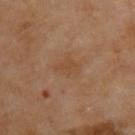Clinical impression:
Imaged during a routine full-body skin examination; the lesion was not biopsied and no histopathology is available.
Background:
Captured under cross-polarized illumination. A male patient, aged 68 to 72. Located on the upper back. The lesion-visualizer software estimated a border-irregularity index near 3/10, internal color variation of about 1.5 on a 0–10 scale, and radial color variation of about 0.5. This image is a 15 mm lesion crop taken from a total-body photograph. The recorded lesion diameter is about 3 mm.